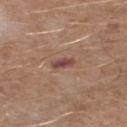From the right lower leg.
A roughly 15 mm field-of-view crop from a total-body skin photograph.
The patient is a male in their mid-60s.